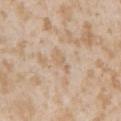workup: catalogued during a skin exam; not biopsied | size: ~3 mm (longest diameter) | anatomic site: the left upper arm | patient: female, in their mid-20s | imaging modality: ~15 mm crop, total-body skin-cancer survey.